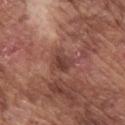Case summary:
* biopsy status: no biopsy performed (imaged during a skin exam)
* image source: 15 mm crop, total-body photography
* automated lesion analysis: a footprint of about 5.5 mm² and a shape eccentricity near 0.55
* patient: male, in their mid- to late 70s
* anatomic site: the right upper arm
* lighting: white-light illumination